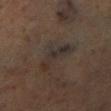Case summary:
• notes · imaged on a skin check; not biopsied
• subject · female, aged approximately 50
• body site · the left lower leg
• automated lesion analysis · an area of roughly 7 mm² and a shape-asymmetry score of about 0.5 (0 = symmetric); a lesion–skin lightness drop of about 6 and a normalized border contrast of about 7.5
• image · total-body-photography crop, ~15 mm field of view
• diameter · ≈4.5 mm